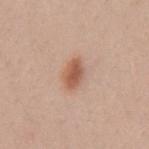biopsy status = imaged on a skin check; not biopsied | diameter = ~3 mm (longest diameter) | acquisition = ~15 mm tile from a whole-body skin photo | location = the arm | automated lesion analysis = an area of roughly 5.5 mm², a shape eccentricity near 0.8, and a symmetry-axis asymmetry near 0.2 | patient = female, aged 23–27 | tile lighting = white-light.